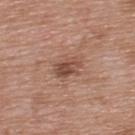No biopsy was performed on this lesion — it was imaged during a full skin examination and was not determined to be concerning.
This is a white-light tile.
A 15 mm close-up tile from a total-body photography series done for melanoma screening.
On the back.
Automated image analysis of the tile measured border irregularity of about 2.5 on a 0–10 scale, a color-variation rating of about 4.5/10, and radial color variation of about 1.5. And it measured a detector confidence of about 100 out of 100 that the crop contains a lesion.
A male subject in their 50s.
Measured at roughly 3 mm in maximum diameter.